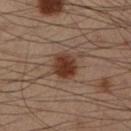Captured during whole-body skin photography for melanoma surveillance; the lesion was not biopsied.